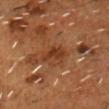The lesion was tiled from a total-body skin photograph and was not biopsied. A male patient, in their mid- to late 50s. Located on the head or neck. Approximately 3 mm at its widest. A 15 mm crop from a total-body photograph taken for skin-cancer surveillance. Automated image analysis of the tile measured a border-irregularity index near 4.5/10. It also reported a detector confidence of about 95 out of 100 that the crop contains a lesion.